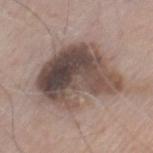The lesion was tiled from a total-body skin photograph and was not biopsied. A male subject in their 80s. On the chest. A close-up tile cropped from a whole-body skin photograph, about 15 mm across.